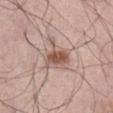Q: Was a biopsy performed?
A: imaged on a skin check; not biopsied
Q: What is the lesion's diameter?
A: about 4.5 mm
Q: What is the imaging modality?
A: total-body-photography crop, ~15 mm field of view
Q: Where on the body is the lesion?
A: the abdomen
Q: Patient demographics?
A: male, aged around 65
Q: How was the tile lit?
A: white-light